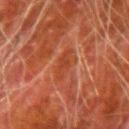Q: Is there a histopathology result?
A: catalogued during a skin exam; not biopsied
Q: Who is the patient?
A: male, approximately 80 years of age
Q: What is the imaging modality?
A: total-body-photography crop, ~15 mm field of view
Q: What is the anatomic site?
A: the right upper arm